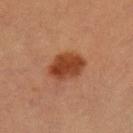Imaged with cross-polarized lighting. Measured at roughly 4.5 mm in maximum diameter. An algorithmic analysis of the crop reported a border-irregularity rating of about 2/10, a color-variation rating of about 3.5/10, and radial color variation of about 1. The patient is a female approximately 25 years of age. This image is a 15 mm lesion crop taken from a total-body photograph. Located on the leg.